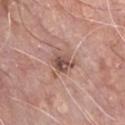The lesion was tiled from a total-body skin photograph and was not biopsied. A 15 mm close-up tile from a total-body photography series done for melanoma screening. The lesion is located on the chest. The patient is a male aged around 60.